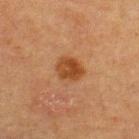Captured during whole-body skin photography for melanoma surveillance; the lesion was not biopsied. This is a cross-polarized tile. The lesion's longest dimension is about 3.5 mm. A male subject, roughly 35 years of age. The lesion is located on the upper back. A close-up tile cropped from a whole-body skin photograph, about 15 mm across.